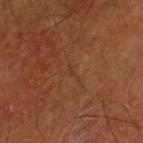workup: catalogued during a skin exam; not biopsied
subject: male, roughly 60 years of age
site: the head or neck
image source: ~15 mm crop, total-body skin-cancer survey